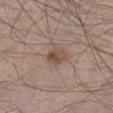Findings:
* biopsy status · no biopsy performed (imaged during a skin exam)
* image source · 15 mm crop, total-body photography
* subject · male, aged approximately 45
* location · the left lower leg
* automated metrics · an area of roughly 5.5 mm², an eccentricity of roughly 0.75, and a symmetry-axis asymmetry near 0.25; a mean CIELAB color near L≈49 a*≈16 b*≈25, about 9 CIELAB-L* units darker than the surrounding skin, and a normalized border contrast of about 7